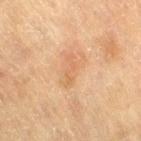- workup · imaged on a skin check; not biopsied
- acquisition · ~15 mm tile from a whole-body skin photo
- image-analysis metrics · a shape eccentricity near 0.95 and a symmetry-axis asymmetry near 0.5; a mean CIELAB color near L≈55 a*≈19 b*≈34 and a lesion-to-skin contrast of about 5 (normalized; higher = more distinct); a border-irregularity index near 7/10 and a color-variation rating of about 0/10; a lesion-detection confidence of about 100/100
- diameter · ~3.5 mm (longest diameter)
- location · the leg
- lighting · cross-polarized
- subject · female, aged approximately 80